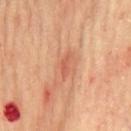The lesion was tiled from a total-body skin photograph and was not biopsied. Approximately 3 mm at its widest. The lesion is on the chest. A male subject, aged around 65. The tile uses cross-polarized illumination. A close-up tile cropped from a whole-body skin photograph, about 15 mm across. An algorithmic analysis of the crop reported an average lesion color of about L≈57 a*≈29 b*≈34 (CIELAB), a lesion–skin lightness drop of about 9, and a normalized lesion–skin contrast near 6. The software also gave border irregularity of about 4 on a 0–10 scale and a color-variation rating of about 0/10. And it measured an automated nevus-likeness rating near 0 out of 100 and a lesion-detection confidence of about 100/100.